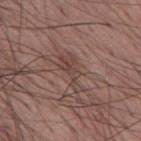{"biopsy_status": "not biopsied; imaged during a skin examination", "lesion_size": {"long_diameter_mm_approx": 5.0}, "patient": {"sex": "male", "age_approx": 55}, "site": "mid back", "image": {"source": "total-body photography crop", "field_of_view_mm": 15}, "lighting": "white-light"}